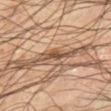Captured during whole-body skin photography for melanoma surveillance; the lesion was not biopsied. Located on the right lower leg. The recorded lesion diameter is about 2.5 mm. A male patient, in their mid-40s. The lesion-visualizer software estimated a lesion area of about 2.5 mm², a shape eccentricity near 0.9, and two-axis asymmetry of about 0.4. The analysis additionally found internal color variation of about 0 on a 0–10 scale and radial color variation of about 0. And it measured an automated nevus-likeness rating near 55 out of 100 and a detector confidence of about 60 out of 100 that the crop contains a lesion. The tile uses cross-polarized illumination. A 15 mm close-up tile from a total-body photography series done for melanoma screening.